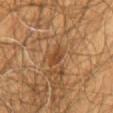| key | value |
|---|---|
| biopsy status | no biopsy performed (imaged during a skin exam) |
| size | ~2.5 mm (longest diameter) |
| patient | male, about 60 years old |
| body site | the chest |
| imaging modality | total-body-photography crop, ~15 mm field of view |
| tile lighting | cross-polarized illumination |
| automated metrics | a border-irregularity rating of about 3/10, internal color variation of about 3.5 on a 0–10 scale, and peripheral color asymmetry of about 1.5; an automated nevus-likeness rating near 10 out of 100 |